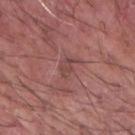Notes:
• biopsy status: imaged on a skin check; not biopsied
• subject: male, aged approximately 55
• site: the right forearm
• acquisition: 15 mm crop, total-body photography
• automated metrics: an area of roughly 3 mm², an outline eccentricity of about 0.8 (0 = round, 1 = elongated), and two-axis asymmetry of about 0.35; an average lesion color of about L≈45 a*≈22 b*≈21 (CIELAB), roughly 6 lightness units darker than nearby skin, and a lesion-to-skin contrast of about 5 (normalized; higher = more distinct); peripheral color asymmetry of about 0.5; a nevus-likeness score of about 0/100